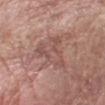Assessment:
Imaged during a routine full-body skin examination; the lesion was not biopsied and no histopathology is available.
Image and clinical context:
From the left forearm. A 15 mm close-up extracted from a 3D total-body photography capture. A female patient aged 58 to 62.